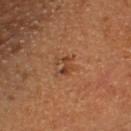Clinical impression: This lesion was catalogued during total-body skin photography and was not selected for biopsy. Acquisition and patient details: The tile uses cross-polarized illumination. The lesion is on the head or neck. This image is a 15 mm lesion crop taken from a total-body photograph. An algorithmic analysis of the crop reported a lesion color around L≈42 a*≈23 b*≈33 in CIELAB, about 8 CIELAB-L* units darker than the surrounding skin, and a lesion-to-skin contrast of about 6.5 (normalized; higher = more distinct). The analysis additionally found a border-irregularity index near 4.5/10 and internal color variation of about 2 on a 0–10 scale. A female subject in their 40s.